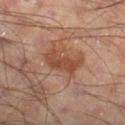The lesion was photographed on a routine skin check and not biopsied; there is no pathology result. The patient is a male aged approximately 55. A 15 mm crop from a total-body photograph taken for skin-cancer surveillance. Automated tile analysis of the lesion measured a lesion area of about 8 mm², a shape eccentricity near 0.9, and a symmetry-axis asymmetry near 0.3. And it measured a mean CIELAB color near L≈43 a*≈22 b*≈30, roughly 9 lightness units darker than nearby skin, and a lesion-to-skin contrast of about 7.5 (normalized; higher = more distinct). The analysis additionally found a nevus-likeness score of about 5/100. The recorded lesion diameter is about 5 mm. Captured under cross-polarized illumination. The lesion is located on the right lower leg.